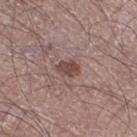Q: Is there a histopathology result?
A: no biopsy performed (imaged during a skin exam)
Q: Lesion size?
A: ~2.5 mm (longest diameter)
Q: Illumination type?
A: white-light illumination
Q: Lesion location?
A: the right thigh
Q: What kind of image is this?
A: ~15 mm crop, total-body skin-cancer survey
Q: What are the patient's age and sex?
A: male, aged around 65
Q: What did automated image analysis measure?
A: a lesion color around L≈45 a*≈20 b*≈22 in CIELAB, a lesion–skin lightness drop of about 10, and a normalized border contrast of about 8